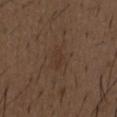Clinical impression: Imaged during a routine full-body skin examination; the lesion was not biopsied and no histopathology is available. Image and clinical context: On the chest. The lesion-visualizer software estimated a footprint of about 2.5 mm², an eccentricity of roughly 0.9, and two-axis asymmetry of about 0.4. It also reported an average lesion color of about L≈33 a*≈16 b*≈24 (CIELAB) and about 5 CIELAB-L* units darker than the surrounding skin. It also reported an automated nevus-likeness rating near 0 out of 100 and a lesion-detection confidence of about 100/100. The subject is a male approximately 50 years of age. A 15 mm close-up extracted from a 3D total-body photography capture. Approximately 2.5 mm at its widest. The tile uses white-light illumination.